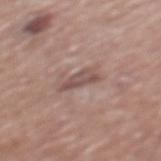Part of a total-body skin-imaging series; this lesion was reviewed on a skin check and was not flagged for biopsy. The recorded lesion diameter is about 3.5 mm. A male patient, aged approximately 75. A lesion tile, about 15 mm wide, cut from a 3D total-body photograph. The lesion is located on the mid back. Automated tile analysis of the lesion measured a border-irregularity index near 4/10, a color-variation rating of about 3/10, and a peripheral color-asymmetry measure near 1. Captured under white-light illumination.